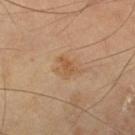The lesion was photographed on a routine skin check and not biopsied; there is no pathology result.
Located on the right thigh.
A male subject, in their mid-60s.
About 3 mm across.
A close-up tile cropped from a whole-body skin photograph, about 15 mm across.